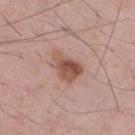The lesion was photographed on a routine skin check and not biopsied; there is no pathology result.
About 4 mm across.
This is a white-light tile.
The lesion is located on the right thigh.
The subject is a male roughly 55 years of age.
A roughly 15 mm field-of-view crop from a total-body skin photograph.